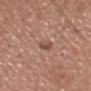Assessment: Imaged during a routine full-body skin examination; the lesion was not biopsied and no histopathology is available. Image and clinical context: Automated image analysis of the tile measured a mean CIELAB color near L≈53 a*≈19 b*≈25, about 7 CIELAB-L* units darker than the surrounding skin, and a normalized lesion–skin contrast near 5. The software also gave a border-irregularity index near 2/10, a within-lesion color-variation index near 4.5/10, and peripheral color asymmetry of about 1.5. And it measured an automated nevus-likeness rating near 0 out of 100 and a lesion-detection confidence of about 100/100. A lesion tile, about 15 mm wide, cut from a 3D total-body photograph. The lesion is located on the head or neck. This is a white-light tile. About 3 mm across. A male patient, aged approximately 40.